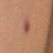Findings:
- follow-up: total-body-photography surveillance lesion; no biopsy
- TBP lesion metrics: a lesion color around L≈40 a*≈20 b*≈23 in CIELAB, about 7 CIELAB-L* units darker than the surrounding skin, and a normalized lesion–skin contrast near 7; lesion-presence confidence of about 100/100
- image: ~15 mm tile from a whole-body skin photo
- lighting: cross-polarized illumination
- subject: female, aged around 40
- body site: the chest
- size: about 4.5 mm